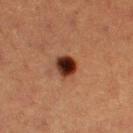{"biopsy_status": "not biopsied; imaged during a skin examination", "lesion_size": {"long_diameter_mm_approx": 3.0}, "patient": {"sex": "female", "age_approx": 40}, "lighting": "cross-polarized", "image": {"source": "total-body photography crop", "field_of_view_mm": 15}, "site": "right thigh"}